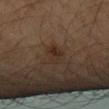The lesion was tiled from a total-body skin photograph and was not biopsied. A female patient, aged 68–72. Automated tile analysis of the lesion measured a lesion area of about 4 mm², a shape eccentricity near 0.75, and a shape-asymmetry score of about 0.3 (0 = symmetric). The software also gave an average lesion color of about L≈24 a*≈13 b*≈21 (CIELAB) and about 5 CIELAB-L* units darker than the surrounding skin. It also reported a lesion-detection confidence of about 100/100. The lesion's longest dimension is about 2.5 mm. A 15 mm close-up tile from a total-body photography series done for melanoma screening. Imaged with cross-polarized lighting. The lesion is located on the left forearm.